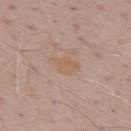workup=catalogued during a skin exam; not biopsied
imaging modality=~15 mm tile from a whole-body skin photo
diameter=about 3.5 mm
subject=male, about 70 years old
anatomic site=the upper back
lighting=white-light illumination
automated lesion analysis=an average lesion color of about L≈59 a*≈17 b*≈30 (CIELAB), a lesion–skin lightness drop of about 5, and a normalized border contrast of about 6; a nevus-likeness score of about 0/100